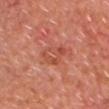biopsy status: imaged on a skin check; not biopsied
diameter: ≈3 mm
site: the upper back
illumination: cross-polarized illumination
automated metrics: an outline eccentricity of about 0.55 (0 = round, 1 = elongated) and a shape-asymmetry score of about 0.35 (0 = symmetric); a border-irregularity rating of about 3.5/10, internal color variation of about 4.5 on a 0–10 scale, and radial color variation of about 1.5; a classifier nevus-likeness of about 0/100 and lesion-presence confidence of about 100/100
image: ~15 mm tile from a whole-body skin photo
subject: male, aged around 60